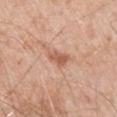<record>
  <biopsy_status>not biopsied; imaged during a skin examination</biopsy_status>
  <image>
    <source>total-body photography crop</source>
    <field_of_view_mm>15</field_of_view_mm>
  </image>
  <site>left upper arm</site>
  <lesion_size>
    <long_diameter_mm_approx>2.5</long_diameter_mm_approx>
  </lesion_size>
  <patient>
    <sex>male</sex>
    <age_approx>55</age_approx>
  </patient>
  <automated_metrics>
    <vs_skin_contrast_norm>7.5</vs_skin_contrast_norm>
  </automated_metrics>
  <lighting>white-light</lighting>
</record>